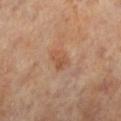Findings:
* follow-up: imaged on a skin check; not biopsied
* lesion size: about 3 mm
* body site: the right leg
* image source: ~15 mm tile from a whole-body skin photo
* patient: female, aged around 65